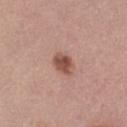No biopsy was performed on this lesion — it was imaged during a full skin examination and was not determined to be concerning. A female subject, in their 50s. The tile uses white-light illumination. About 3 mm across. Cropped from a total-body skin-imaging series; the visible field is about 15 mm. From the left thigh.